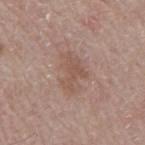Findings:
- biopsy status: total-body-photography surveillance lesion; no biopsy
- image: total-body-photography crop, ~15 mm field of view
- diameter: about 4.5 mm
- subject: male, approximately 65 years of age
- illumination: white-light illumination
- body site: the back